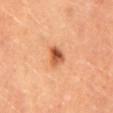biopsy status: imaged on a skin check; not biopsied
size: about 2.5 mm
subject: female, about 30 years old
location: the mid back
image source: 15 mm crop, total-body photography